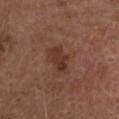Imaged during a routine full-body skin examination; the lesion was not biopsied and no histopathology is available. An algorithmic analysis of the crop reported a classifier nevus-likeness of about 10/100 and a detector confidence of about 100 out of 100 that the crop contains a lesion. From the head or neck. Cropped from a total-body skin-imaging series; the visible field is about 15 mm. Approximately 3.5 mm at its widest. Imaged with white-light lighting. A male subject, aged 73 to 77.